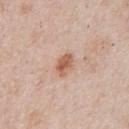Impression: No biopsy was performed on this lesion — it was imaged during a full skin examination and was not determined to be concerning.